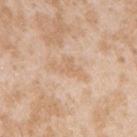The lesion was photographed on a routine skin check and not biopsied; there is no pathology result. This is a white-light tile. Located on the right upper arm. A female patient aged approximately 25. A 15 mm close-up tile from a total-body photography series done for melanoma screening.